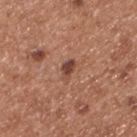Image and clinical context:
A 15 mm crop from a total-body photograph taken for skin-cancer surveillance. On the upper back. About 2.5 mm across. The total-body-photography lesion software estimated a shape eccentricity near 0.85 and two-axis asymmetry of about 0.3. It also reported roughly 12 lightness units darker than nearby skin. The patient is a male aged 53–57.